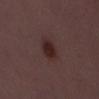Assessment: This lesion was catalogued during total-body skin photography and was not selected for biopsy. Image and clinical context: A 15 mm close-up tile from a total-body photography series done for melanoma screening. On the left thigh. The patient is a female roughly 30 years of age.